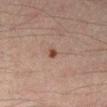Assessment:
Part of a total-body skin-imaging series; this lesion was reviewed on a skin check and was not flagged for biopsy.